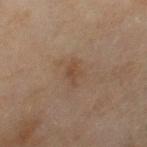follow-up = imaged on a skin check; not biopsied
image = 15 mm crop, total-body photography
body site = the leg
patient = female, roughly 60 years of age